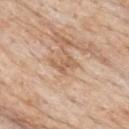Assessment:
The lesion was photographed on a routine skin check and not biopsied; there is no pathology result.
Context:
Cropped from a total-body skin-imaging series; the visible field is about 15 mm. A male patient aged approximately 85 or older. Located on the upper back.